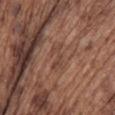The lesion was photographed on a routine skin check and not biopsied; there is no pathology result. Captured under white-light illumination. A male patient in their mid-70s. Longest diameter approximately 3 mm. Cropped from a whole-body photographic skin survey; the tile spans about 15 mm. Located on the upper back. An algorithmic analysis of the crop reported a lesion area of about 4 mm² and two-axis asymmetry of about 0.55. It also reported an average lesion color of about L≈44 a*≈20 b*≈26 (CIELAB) and roughly 6 lightness units darker than nearby skin. It also reported an automated nevus-likeness rating near 0 out of 100 and a lesion-detection confidence of about 85/100.